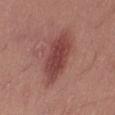biopsy_status: not biopsied; imaged during a skin examination
image:
  source: total-body photography crop
  field_of_view_mm: 15
automated_metrics:
  cielab_L: 44
  cielab_a: 26
  cielab_b: 23
  vs_skin_contrast_norm: 8.5
  color_variation_0_10: 3.5
lesion_size:
  long_diameter_mm_approx: 7.5
patient:
  sex: female
  age_approx: 40
site: leg
lighting: white-light